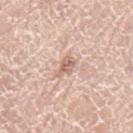{
  "biopsy_status": "not biopsied; imaged during a skin examination",
  "patient": {
    "sex": "male",
    "age_approx": 75
  },
  "site": "left upper arm",
  "lesion_size": {
    "long_diameter_mm_approx": 2.5
  },
  "image": {
    "source": "total-body photography crop",
    "field_of_view_mm": 15
  },
  "automated_metrics": {
    "shape_asymmetry": 0.45,
    "nevus_likeness_0_100": 0,
    "lesion_detection_confidence_0_100": 100
  },
  "lighting": "white-light"
}